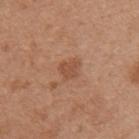Q: Was a biopsy performed?
A: catalogued during a skin exam; not biopsied
Q: What is the anatomic site?
A: the mid back
Q: How was the tile lit?
A: white-light
Q: What kind of image is this?
A: total-body-photography crop, ~15 mm field of view
Q: Patient demographics?
A: female, in their 40s